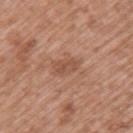| feature | finding |
|---|---|
| follow-up | imaged on a skin check; not biopsied |
| patient | male, aged 48 to 52 |
| illumination | white-light illumination |
| TBP lesion metrics | a mean CIELAB color near L≈51 a*≈22 b*≈30, a lesion–skin lightness drop of about 8, and a lesion-to-skin contrast of about 5.5 (normalized; higher = more distinct); internal color variation of about 2.5 on a 0–10 scale and peripheral color asymmetry of about 1; a classifier nevus-likeness of about 0/100 |
| body site | the back |
| image source | total-body-photography crop, ~15 mm field of view |